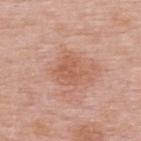Imaged during a routine full-body skin examination; the lesion was not biopsied and no histopathology is available. Automated image analysis of the tile measured a lesion color around L≈58 a*≈24 b*≈32 in CIELAB, about 7 CIELAB-L* units darker than the surrounding skin, and a normalized border contrast of about 5.5. And it measured a detector confidence of about 100 out of 100 that the crop contains a lesion. Captured under white-light illumination. The lesion is located on the upper back. A lesion tile, about 15 mm wide, cut from a 3D total-body photograph. The patient is a female aged 48–52.